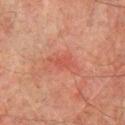The lesion was tiled from a total-body skin photograph and was not biopsied. Located on the chest. A male subject, aged 73 to 77. This is a cross-polarized tile. The lesion's longest dimension is about 2.5 mm. A 15 mm close-up tile from a total-body photography series done for melanoma screening.